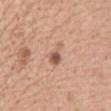Assessment:
Imaged during a routine full-body skin examination; the lesion was not biopsied and no histopathology is available.
Clinical summary:
This is a white-light tile. A female subject, aged around 55. A 15 mm crop from a total-body photograph taken for skin-cancer surveillance. The lesion's longest dimension is about 3 mm. From the abdomen.